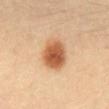The lesion was photographed on a routine skin check and not biopsied; there is no pathology result. The patient is a male approximately 20 years of age. The tile uses cross-polarized illumination. From the abdomen. The recorded lesion diameter is about 4 mm. A close-up tile cropped from a whole-body skin photograph, about 15 mm across.